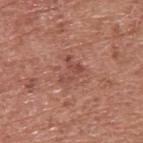| key | value |
|---|---|
| follow-up | no biopsy performed (imaged during a skin exam) |
| location | the back |
| size | ~3 mm (longest diameter) |
| patient | male, in their mid- to late 70s |
| image | ~15 mm crop, total-body skin-cancer survey |